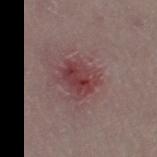Q: Was a biopsy performed?
A: no biopsy performed (imaged during a skin exam)
Q: What lighting was used for the tile?
A: white-light
Q: What is the imaging modality?
A: ~15 mm crop, total-body skin-cancer survey
Q: What is the lesion's diameter?
A: ≈4 mm
Q: Who is the patient?
A: female, aged around 30
Q: What did automated image analysis measure?
A: an eccentricity of roughly 0.6 and a symmetry-axis asymmetry near 0.25
Q: Where on the body is the lesion?
A: the right thigh